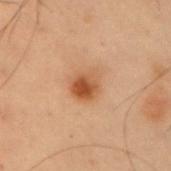follow-up = no biopsy performed (imaged during a skin exam)
tile lighting = cross-polarized
site = the right upper arm
image source = 15 mm crop, total-body photography
subject = male, approximately 55 years of age
diameter = ≈3.5 mm